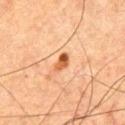From the chest.
Cropped from a whole-body photographic skin survey; the tile spans about 15 mm.
A male subject in their mid-60s.
About 2.5 mm across.